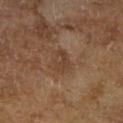{"biopsy_status": "not biopsied; imaged during a skin examination", "lighting": "cross-polarized", "image": {"source": "total-body photography crop", "field_of_view_mm": 15}, "patient": {"sex": "male", "age_approx": 65}, "lesion_size": {"long_diameter_mm_approx": 3.0}}